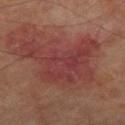No biopsy was performed on this lesion — it was imaged during a full skin examination and was not determined to be concerning. The lesion is on the leg. Cropped from a total-body skin-imaging series; the visible field is about 15 mm. The subject is a male roughly 70 years of age.